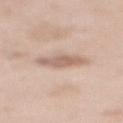Clinical summary: Located on the upper back. The patient is a female aged 63 to 67. Longest diameter approximately 4.5 mm. A lesion tile, about 15 mm wide, cut from a 3D total-body photograph.